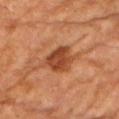| feature | finding |
|---|---|
| notes | no biopsy performed (imaged during a skin exam) |
| image source | total-body-photography crop, ~15 mm field of view |
| subject | male, aged approximately 60 |
| image-analysis metrics | a lesion area of about 11 mm²; a color-variation rating of about 4/10; a nevus-likeness score of about 60/100 and a lesion-detection confidence of about 100/100 |
| body site | the left arm |